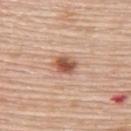workup=no biopsy performed (imaged during a skin exam) | site=the upper back | tile lighting=white-light | subject=female, in their mid- to late 60s | image source=~15 mm crop, total-body skin-cancer survey.